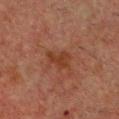Clinical impression:
Recorded during total-body skin imaging; not selected for excision or biopsy.
Acquisition and patient details:
The lesion is located on the chest. A male subject, aged 48–52. Cropped from a total-body skin-imaging series; the visible field is about 15 mm. Automated tile analysis of the lesion measured a lesion area of about 6 mm² and an outline eccentricity of about 0.8 (0 = round, 1 = elongated). Longest diameter approximately 3.5 mm.